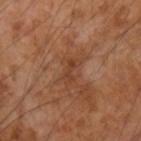{"biopsy_status": "not biopsied; imaged during a skin examination", "automated_metrics": {"cielab_L": 41, "cielab_a": 23, "cielab_b": 32, "vs_skin_contrast_norm": 5.5}, "image": {"source": "total-body photography crop", "field_of_view_mm": 15}, "patient": {"sex": "male", "age_approx": 55}, "site": "left forearm", "lesion_size": {"long_diameter_mm_approx": 2.5}, "lighting": "cross-polarized"}